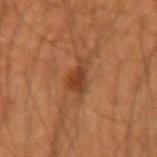| feature | finding |
|---|---|
| biopsy status | catalogued during a skin exam; not biopsied |
| patient | male, about 55 years old |
| body site | the left forearm |
| illumination | cross-polarized |
| acquisition | ~15 mm crop, total-body skin-cancer survey |
| lesion size | ≈4.5 mm |
| automated lesion analysis | a footprint of about 7 mm² and a shape-asymmetry score of about 0.45 (0 = symmetric); a mean CIELAB color near L≈35 a*≈21 b*≈31 and a normalized border contrast of about 7.5; border irregularity of about 6 on a 0–10 scale; a detector confidence of about 100 out of 100 that the crop contains a lesion |